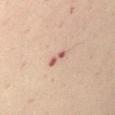• notes: catalogued during a skin exam; not biopsied
• size: about 2.5 mm
• patient: male, about 50 years old
• anatomic site: the left upper arm
• TBP lesion metrics: a footprint of about 2.5 mm² and an eccentricity of roughly 0.95
• image: ~15 mm crop, total-body skin-cancer survey
• tile lighting: cross-polarized illumination